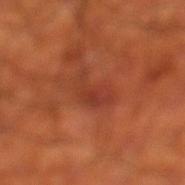The lesion is located on the leg.
Imaged with cross-polarized lighting.
A male patient aged approximately 70.
A region of skin cropped from a whole-body photographic capture, roughly 15 mm wide.
The recorded lesion diameter is about 4.5 mm.
An algorithmic analysis of the crop reported a footprint of about 6.5 mm², a shape eccentricity near 0.85, and a symmetry-axis asymmetry near 0.45. The analysis additionally found an average lesion color of about L≈38 a*≈30 b*≈32 (CIELAB) and a lesion-to-skin contrast of about 5.5 (normalized; higher = more distinct). It also reported a border-irregularity index near 5/10, a color-variation rating of about 3/10, and radial color variation of about 1.